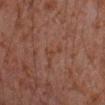The lesion was photographed on a routine skin check and not biopsied; there is no pathology result.
An algorithmic analysis of the crop reported a lesion area of about 3 mm² and a shape eccentricity near 0.9. It also reported a classifier nevus-likeness of about 0/100 and a lesion-detection confidence of about 100/100.
A 15 mm close-up extracted from a 3D total-body photography capture.
A male subject roughly 30 years of age.
The lesion is located on the right forearm.
Captured under cross-polarized illumination.
The recorded lesion diameter is about 3 mm.